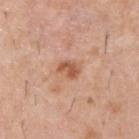Findings:
- anatomic site · the upper back
- patient · male, in their 60s
- lighting · white-light illumination
- acquisition · ~15 mm crop, total-body skin-cancer survey
- lesion diameter · ≈2.5 mm